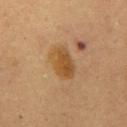The lesion is on the mid back. Imaged with cross-polarized lighting. A female patient approximately 60 years of age. A close-up tile cropped from a whole-body skin photograph, about 15 mm across. Approximately 4.5 mm at its widest. Automated image analysis of the tile measured an average lesion color of about L≈45 a*≈17 b*≈35 (CIELAB), roughly 8 lightness units darker than nearby skin, and a normalized lesion–skin contrast near 7.5.